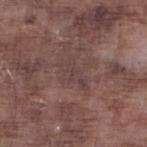Impression: Part of a total-body skin-imaging series; this lesion was reviewed on a skin check and was not flagged for biopsy. Image and clinical context: A roughly 15 mm field-of-view crop from a total-body skin photograph. On the left lower leg. Longest diameter approximately 4 mm. A male subject aged around 75. Automated tile analysis of the lesion measured an automated nevus-likeness rating near 0 out of 100 and a detector confidence of about 55 out of 100 that the crop contains a lesion.